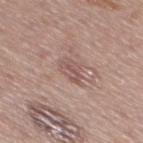follow-up — no biopsy performed (imaged during a skin exam).